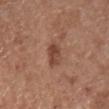The lesion was tiled from a total-body skin photograph and was not biopsied. Imaged with white-light lighting. Longest diameter approximately 3 mm. From the chest. A male subject about 55 years old. A roughly 15 mm field-of-view crop from a total-body skin photograph.